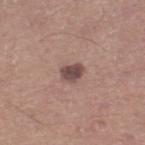Clinical impression:
Part of a total-body skin-imaging series; this lesion was reviewed on a skin check and was not flagged for biopsy.
Background:
This is a white-light tile. The lesion is located on the right lower leg. A 15 mm crop from a total-body photograph taken for skin-cancer surveillance. The recorded lesion diameter is about 2.5 mm. A male patient, aged 43 to 47. Automated tile analysis of the lesion measured a footprint of about 4.5 mm², a shape eccentricity near 0.6, and a shape-asymmetry score of about 0.25 (0 = symmetric). It also reported a lesion color around L≈47 a*≈18 b*≈20 in CIELAB, roughly 13 lightness units darker than nearby skin, and a normalized lesion–skin contrast near 10. The software also gave border irregularity of about 2.5 on a 0–10 scale, internal color variation of about 2.5 on a 0–10 scale, and radial color variation of about 1. The analysis additionally found a classifier nevus-likeness of about 20/100.